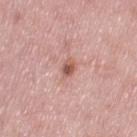| key | value |
|---|---|
| follow-up | imaged on a skin check; not biopsied |
| anatomic site | the left thigh |
| imaging modality | 15 mm crop, total-body photography |
| subject | female, aged around 50 |
| diameter | ~2.5 mm (longest diameter) |
| tile lighting | white-light illumination |